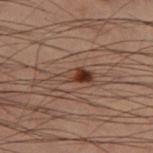The lesion was photographed on a routine skin check and not biopsied; there is no pathology result.
The subject is a male aged 53–57.
The lesion is on the left thigh.
Measured at roughly 4 mm in maximum diameter.
Cropped from a total-body skin-imaging series; the visible field is about 15 mm.
The tile uses cross-polarized illumination.